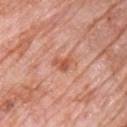follow-up — no biopsy performed (imaged during a skin exam)
lighting — white-light illumination
imaging modality — total-body-photography crop, ~15 mm field of view
patient — male, aged 78–82
automated lesion analysis — a lesion area of about 3.5 mm², a shape eccentricity near 0.75, and two-axis asymmetry of about 0.4; a lesion color around L≈56 a*≈28 b*≈34 in CIELAB
site — the chest
diameter — about 2.5 mm